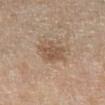The lesion was tiled from a total-body skin photograph and was not biopsied.
The recorded lesion diameter is about 3.5 mm.
A 15 mm close-up extracted from a 3D total-body photography capture.
The subject is a female aged around 60.
From the right lower leg.